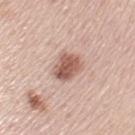Captured during whole-body skin photography for melanoma surveillance; the lesion was not biopsied.
The patient is a female about 60 years old.
Located on the left upper arm.
An algorithmic analysis of the crop reported a lesion area of about 7.5 mm², an eccentricity of roughly 0.7, and two-axis asymmetry of about 0.2. And it measured a lesion–skin lightness drop of about 15. And it measured border irregularity of about 2 on a 0–10 scale, internal color variation of about 4 on a 0–10 scale, and a peripheral color-asymmetry measure near 1.5. The analysis additionally found a classifier nevus-likeness of about 90/100 and lesion-presence confidence of about 100/100.
Captured under white-light illumination.
Cropped from a total-body skin-imaging series; the visible field is about 15 mm.